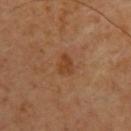notes: total-body-photography surveillance lesion; no biopsy
subject: male, aged 43 to 47
illumination: cross-polarized
anatomic site: the upper back
lesion diameter: ≈2.5 mm
imaging modality: total-body-photography crop, ~15 mm field of view